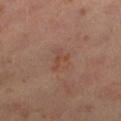follow-up: no biopsy performed (imaged during a skin exam) | imaging modality: total-body-photography crop, ~15 mm field of view | illumination: cross-polarized illumination | body site: the right lower leg | patient: female, aged around 40 | TBP lesion metrics: an area of roughly 5 mm² and an eccentricity of roughly 0.7; a mean CIELAB color near L≈45 a*≈20 b*≈27 and a lesion-to-skin contrast of about 4.5 (normalized; higher = more distinct); an automated nevus-likeness rating near 5 out of 100 and a detector confidence of about 100 out of 100 that the crop contains a lesion.